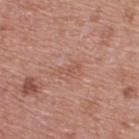Acquisition and patient details: A 15 mm close-up extracted from a 3D total-body photography capture. From the upper back. The patient is a male about 70 years old.